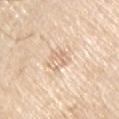Part of a total-body skin-imaging series; this lesion was reviewed on a skin check and was not flagged for biopsy.
From the arm.
A region of skin cropped from a whole-body photographic capture, roughly 15 mm wide.
The total-body-photography lesion software estimated a lesion area of about 5.5 mm², a shape eccentricity near 0.55, and two-axis asymmetry of about 0.3. And it measured an average lesion color of about L≈73 a*≈16 b*≈32 (CIELAB) and a lesion-to-skin contrast of about 4.5 (normalized; higher = more distinct). The analysis additionally found a color-variation rating of about 3.5/10 and radial color variation of about 1.5.
The lesion's longest dimension is about 3 mm.
The patient is a male in their 80s.
The tile uses white-light illumination.